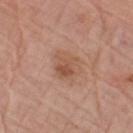Background: Automated tile analysis of the lesion measured a lesion area of about 7.5 mm². The analysis additionally found a border-irregularity index near 2.5/10, a color-variation rating of about 5.5/10, and a peripheral color-asymmetry measure near 2. This image is a 15 mm lesion crop taken from a total-body photograph. The subject is a male aged around 80. The lesion's longest dimension is about 3.5 mm. On the left forearm. This is a white-light tile.